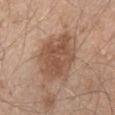Q: Was this lesion biopsied?
A: total-body-photography surveillance lesion; no biopsy
Q: What are the patient's age and sex?
A: male, approximately 45 years of age
Q: Where on the body is the lesion?
A: the back
Q: How was this image acquired?
A: total-body-photography crop, ~15 mm field of view
Q: How large is the lesion?
A: ≈6 mm
Q: What lighting was used for the tile?
A: white-light